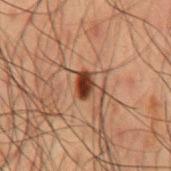Q: Is there a histopathology result?
A: no biopsy performed (imaged during a skin exam)
Q: What is the imaging modality?
A: total-body-photography crop, ~15 mm field of view
Q: Where on the body is the lesion?
A: the mid back
Q: Patient demographics?
A: male, aged 48 to 52